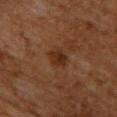Acquisition and patient details: Imaged with cross-polarized lighting. Located on the front of the torso. Measured at roughly 2.5 mm in maximum diameter. A 15 mm crop from a total-body photograph taken for skin-cancer surveillance. A male patient, aged 58–62.